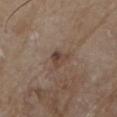Q: Was a biopsy performed?
A: no biopsy performed (imaged during a skin exam)
Q: What is the lesion's diameter?
A: about 2.5 mm
Q: What is the imaging modality?
A: ~15 mm tile from a whole-body skin photo
Q: Automated lesion metrics?
A: an average lesion color of about L≈41 a*≈16 b*≈23 (CIELAB) and roughly 9 lightness units darker than nearby skin; border irregularity of about 4 on a 0–10 scale and internal color variation of about 4 on a 0–10 scale
Q: Illumination type?
A: white-light
Q: Who is the patient?
A: male, approximately 80 years of age
Q: Where on the body is the lesion?
A: the front of the torso